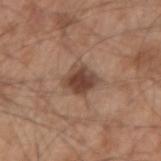Clinical impression: This lesion was catalogued during total-body skin photography and was not selected for biopsy. Background: The lesion's longest dimension is about 3 mm. A male patient, roughly 55 years of age. The lesion is on the right forearm. A region of skin cropped from a whole-body photographic capture, roughly 15 mm wide. Automated tile analysis of the lesion measured a footprint of about 8.5 mm², an outline eccentricity of about 0.45 (0 = round, 1 = elongated), and a shape-asymmetry score of about 0.2 (0 = symmetric). And it measured a lesion color around L≈44 a*≈19 b*≈27 in CIELAB, a lesion–skin lightness drop of about 12, and a normalized border contrast of about 9.5. And it measured border irregularity of about 2.5 on a 0–10 scale, a color-variation rating of about 4/10, and radial color variation of about 1. And it measured an automated nevus-likeness rating near 75 out of 100 and a lesion-detection confidence of about 100/100.